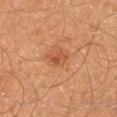<record>
<biopsy_status>not biopsied; imaged during a skin examination</biopsy_status>
<lesion_size>
  <long_diameter_mm_approx>2.5</long_diameter_mm_approx>
</lesion_size>
<site>left lower leg</site>
<image>
  <source>total-body photography crop</source>
  <field_of_view_mm>15</field_of_view_mm>
</image>
<patient>
  <sex>male</sex>
  <age_approx>40</age_approx>
</patient>
</record>